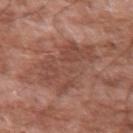Q: Is there a histopathology result?
A: total-body-photography surveillance lesion; no biopsy
Q: Who is the patient?
A: male, aged 58 to 62
Q: What is the imaging modality?
A: 15 mm crop, total-body photography
Q: Lesion location?
A: the left upper arm
Q: What is the lesion's diameter?
A: ≈8 mm
Q: What lighting was used for the tile?
A: white-light illumination